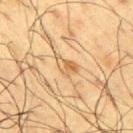notes=catalogued during a skin exam; not biopsied
body site=the right upper arm
image source=15 mm crop, total-body photography
patient=male, aged around 60